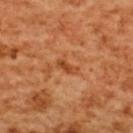The lesion was photographed on a routine skin check and not biopsied; there is no pathology result.
A lesion tile, about 15 mm wide, cut from a 3D total-body photograph.
A female subject about 55 years old.
This is a cross-polarized tile.
Automated tile analysis of the lesion measured a symmetry-axis asymmetry near 0.35. The analysis additionally found an automated nevus-likeness rating near 0 out of 100.
The lesion is on the upper back.
Measured at roughly 2.5 mm in maximum diameter.